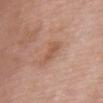Assessment: The lesion was photographed on a routine skin check and not biopsied; there is no pathology result. Acquisition and patient details: The lesion's longest dimension is about 3 mm. Cropped from a whole-body photographic skin survey; the tile spans about 15 mm. A female patient, aged 68 to 72. The lesion is located on the front of the torso.